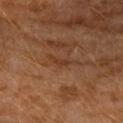Part of a total-body skin-imaging series; this lesion was reviewed on a skin check and was not flagged for biopsy.
Located on the right upper arm.
Automated tile analysis of the lesion measured an outline eccentricity of about 0.95 (0 = round, 1 = elongated) and a symmetry-axis asymmetry near 0.65. And it measured border irregularity of about 7 on a 0–10 scale, a color-variation rating of about 0/10, and peripheral color asymmetry of about 0. The software also gave an automated nevus-likeness rating near 0 out of 100 and a detector confidence of about 60 out of 100 that the crop contains a lesion.
Measured at roughly 3.5 mm in maximum diameter.
Captured under cross-polarized illumination.
A male patient approximately 60 years of age.
A close-up tile cropped from a whole-body skin photograph, about 15 mm across.